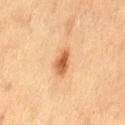notes = no biopsy performed (imaged during a skin exam) | body site = the left thigh | imaging modality = total-body-photography crop, ~15 mm field of view | subject = female, aged approximately 55.